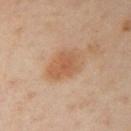biopsy status = total-body-photography surveillance lesion; no biopsy | subject = female, aged around 40 | illumination = cross-polarized | diameter = ~4.5 mm (longest diameter) | image source = total-body-photography crop, ~15 mm field of view | location = the left arm | automated lesion analysis = a mean CIELAB color near L≈59 a*≈21 b*≈35 and a lesion–skin lightness drop of about 9.